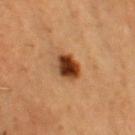This lesion was catalogued during total-body skin photography and was not selected for biopsy. A male subject roughly 50 years of age. From the chest. Cropped from a total-body skin-imaging series; the visible field is about 15 mm.